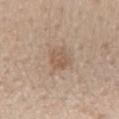{
  "patient": {
    "sex": "male",
    "age_approx": 60
  },
  "lesion_size": {
    "long_diameter_mm_approx": 3.0
  },
  "site": "mid back",
  "automated_metrics": {
    "eccentricity": 0.5,
    "shape_asymmetry": 0.2,
    "color_variation_0_10": 2.5,
    "peripheral_color_asymmetry": 1.0,
    "nevus_likeness_0_100": 20
  },
  "lighting": "white-light",
  "image": {
    "source": "total-body photography crop",
    "field_of_view_mm": 15
  }
}